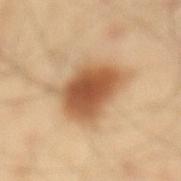Impression: Recorded during total-body skin imaging; not selected for excision or biopsy. Background: Approximately 6 mm at its widest. A 15 mm close-up tile from a total-body photography series done for melanoma screening. From the mid back. This is a cross-polarized tile. A male patient, in their 40s.